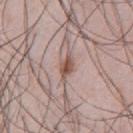Assessment:
Recorded during total-body skin imaging; not selected for excision or biopsy.
Acquisition and patient details:
This image is a 15 mm lesion crop taken from a total-body photograph. The lesion-visualizer software estimated a border-irregularity rating of about 4/10, internal color variation of about 4 on a 0–10 scale, and peripheral color asymmetry of about 1.5. The analysis additionally found an automated nevus-likeness rating near 95 out of 100 and a lesion-detection confidence of about 100/100. The tile uses white-light illumination. The lesion is on the chest. Measured at roughly 3.5 mm in maximum diameter. A male subject approximately 35 years of age.